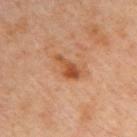Context: Located on the right upper arm. A male subject aged 43 to 47. A lesion tile, about 15 mm wide, cut from a 3D total-body photograph. Automated tile analysis of the lesion measured a footprint of about 5.5 mm² and a shape eccentricity near 0.85. About 4 mm across. Captured under cross-polarized illumination.